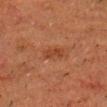biopsy status: no biopsy performed (imaged during a skin exam) | lesion diameter: ≈2.5 mm | image source: total-body-photography crop, ~15 mm field of view | site: the head or neck | automated lesion analysis: a lesion area of about 4 mm², an outline eccentricity of about 0.8 (0 = round, 1 = elongated), and two-axis asymmetry of about 0.25; a lesion color around L≈35 a*≈22 b*≈29 in CIELAB, a lesion–skin lightness drop of about 6, and a lesion-to-skin contrast of about 6 (normalized; higher = more distinct) | patient: male, in their mid-70s | illumination: cross-polarized.